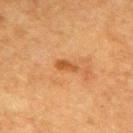Findings:
- biopsy status: no biopsy performed (imaged during a skin exam)
- imaging modality: ~15 mm crop, total-body skin-cancer survey
- anatomic site: the upper back
- patient: female, aged around 55